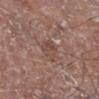Q: What is the lesion's diameter?
A: ~3 mm (longest diameter)
Q: What kind of image is this?
A: ~15 mm tile from a whole-body skin photo
Q: Lesion location?
A: the right lower leg
Q: Automated lesion metrics?
A: a shape eccentricity near 0.65 and a symmetry-axis asymmetry near 0.3; a lesion color around L≈46 a*≈18 b*≈23 in CIELAB, about 7 CIELAB-L* units darker than the surrounding skin, and a normalized lesion–skin contrast near 5.5; a border-irregularity rating of about 3/10, a color-variation rating of about 3/10, and peripheral color asymmetry of about 1; an automated nevus-likeness rating near 0 out of 100 and lesion-presence confidence of about 100/100
Q: What lighting was used for the tile?
A: white-light illumination
Q: What are the patient's age and sex?
A: male, aged 68–72